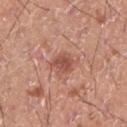Image and clinical context: A 15 mm crop from a total-body photograph taken for skin-cancer surveillance. The recorded lesion diameter is about 3 mm. The lesion-visualizer software estimated a lesion color around L≈52 a*≈25 b*≈28 in CIELAB, roughly 10 lightness units darker than nearby skin, and a normalized lesion–skin contrast near 7. And it measured border irregularity of about 2.5 on a 0–10 scale and a within-lesion color-variation index near 2/10. The patient is a male roughly 20 years of age. Captured under white-light illumination. The lesion is on the leg.